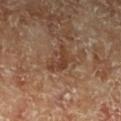Q: What is the lesion's diameter?
A: ~4 mm (longest diameter)
Q: Where on the body is the lesion?
A: the left lower leg
Q: What kind of image is this?
A: ~15 mm crop, total-body skin-cancer survey
Q: What are the patient's age and sex?
A: male, aged approximately 70
Q: What lighting was used for the tile?
A: cross-polarized
Q: Automated lesion metrics?
A: an average lesion color of about L≈38 a*≈20 b*≈29 (CIELAB), roughly 8 lightness units darker than nearby skin, and a normalized border contrast of about 7; a classifier nevus-likeness of about 0/100